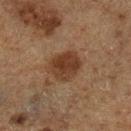Q: Is there a histopathology result?
A: no biopsy performed (imaged during a skin exam)
Q: Patient demographics?
A: male, aged 73–77
Q: Where on the body is the lesion?
A: the left lower leg
Q: What is the imaging modality?
A: total-body-photography crop, ~15 mm field of view